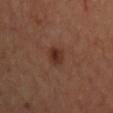Clinical impression: Part of a total-body skin-imaging series; this lesion was reviewed on a skin check and was not flagged for biopsy. Acquisition and patient details: Approximately 2.5 mm at its widest. Located on the chest. Cropped from a total-body skin-imaging series; the visible field is about 15 mm. This is a cross-polarized tile. The subject is a male in their mid- to late 60s.